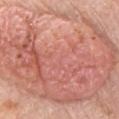Clinical impression: Part of a total-body skin-imaging series; this lesion was reviewed on a skin check and was not flagged for biopsy. Clinical summary: Longest diameter approximately 19 mm. A male patient aged approximately 80. The lesion is on the chest. A roughly 15 mm field-of-view crop from a total-body skin photograph. Automated tile analysis of the lesion measured an area of roughly 165 mm², a shape eccentricity near 0.75, and a symmetry-axis asymmetry near 0.25. It also reported a lesion color around L≈62 a*≈25 b*≈29 in CIELAB, about 9 CIELAB-L* units darker than the surrounding skin, and a normalized lesion–skin contrast near 6. The software also gave a border-irregularity rating of about 5/10. Imaged with white-light lighting.